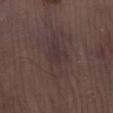biopsy status: no biopsy performed (imaged during a skin exam) | lesion diameter: ≈5.5 mm | patient: male, aged 68–72 | image: total-body-photography crop, ~15 mm field of view | anatomic site: the right lower leg | TBP lesion metrics: a lesion color around L≈32 a*≈13 b*≈15 in CIELAB and about 5 CIELAB-L* units darker than the surrounding skin; internal color variation of about 4 on a 0–10 scale and peripheral color asymmetry of about 1.5 | lighting: white-light illumination.